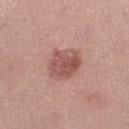Recorded during total-body skin imaging; not selected for excision or biopsy. Automated image analysis of the tile measured a border-irregularity rating of about 2/10, a color-variation rating of about 4.5/10, and a peripheral color-asymmetry measure near 1.5. It also reported a lesion-detection confidence of about 100/100. The patient is a female in their mid-50s. The lesion is located on the leg. Imaged with white-light lighting. Longest diameter approximately 5 mm. This image is a 15 mm lesion crop taken from a total-body photograph.